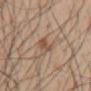Captured during whole-body skin photography for melanoma surveillance; the lesion was not biopsied.
A male patient, approximately 55 years of age.
An algorithmic analysis of the crop reported an area of roughly 4.5 mm² and an outline eccentricity of about 0.65 (0 = round, 1 = elongated). And it measured an average lesion color of about L≈52 a*≈18 b*≈28 (CIELAB), roughly 9 lightness units darker than nearby skin, and a normalized lesion–skin contrast near 6.5.
Measured at roughly 3 mm in maximum diameter.
The lesion is on the back.
A 15 mm close-up tile from a total-body photography series done for melanoma screening.